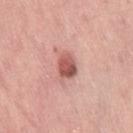subject: female, aged around 45 | tile lighting: white-light illumination | anatomic site: the right thigh | automated metrics: a footprint of about 6 mm², a shape eccentricity near 0.7, and a symmetry-axis asymmetry near 0.15; border irregularity of about 1.5 on a 0–10 scale | lesion size: ~3 mm (longest diameter) | imaging modality: 15 mm crop, total-body photography.